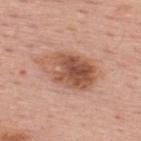The lesion's longest dimension is about 7 mm.
Automated image analysis of the tile measured an outline eccentricity of about 0.8 (0 = round, 1 = elongated) and a shape-asymmetry score of about 0.15 (0 = symmetric). The software also gave a border-irregularity rating of about 2/10.
The lesion is on the upper back.
A male subject, aged approximately 65.
Cropped from a whole-body photographic skin survey; the tile spans about 15 mm.
Captured under white-light illumination.